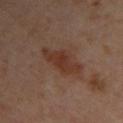Q: Was a biopsy performed?
A: total-body-photography surveillance lesion; no biopsy
Q: Lesion location?
A: the back
Q: What kind of image is this?
A: 15 mm crop, total-body photography
Q: Who is the patient?
A: female, aged approximately 40